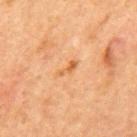follow-up=no biopsy performed (imaged during a skin exam) | imaging modality=~15 mm crop, total-body skin-cancer survey | subject=male, in their mid-60s | anatomic site=the mid back | diameter=~2.5 mm (longest diameter) | tile lighting=cross-polarized.